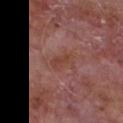follow-up: imaged on a skin check; not biopsied | image source: ~15 mm crop, total-body skin-cancer survey | subject: male, aged 63–67 | image-analysis metrics: a footprint of about 4.5 mm², a shape eccentricity near 0.85, and a shape-asymmetry score of about 0.25 (0 = symmetric); an average lesion color of about L≈43 a*≈23 b*≈26 (CIELAB) and a normalized border contrast of about 6 | tile lighting: white-light illumination | size: about 3 mm | site: the front of the torso.